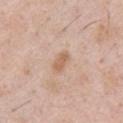The subject is a male in their 50s.
From the chest.
The lesion-visualizer software estimated an average lesion color of about L≈62 a*≈19 b*≈32 (CIELAB) and a lesion–skin lightness drop of about 9. It also reported border irregularity of about 2.5 on a 0–10 scale, internal color variation of about 1 on a 0–10 scale, and a peripheral color-asymmetry measure near 0.5. The analysis additionally found a classifier nevus-likeness of about 50/100.
The tile uses white-light illumination.
A roughly 15 mm field-of-view crop from a total-body skin photograph.